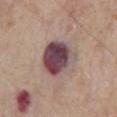Part of a total-body skin-imaging series; this lesion was reviewed on a skin check and was not flagged for biopsy. The lesion is located on the front of the torso. A male subject aged around 65. Cropped from a total-body skin-imaging series; the visible field is about 15 mm. Captured under white-light illumination. About 5.5 mm across.